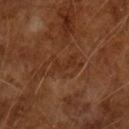Impression:
Part of a total-body skin-imaging series; this lesion was reviewed on a skin check and was not flagged for biopsy.
Image and clinical context:
Cropped from a whole-body photographic skin survey; the tile spans about 15 mm. Automated image analysis of the tile measured a footprint of about 3 mm² and a shape eccentricity near 0.9. The software also gave an automated nevus-likeness rating near 0 out of 100 and lesion-presence confidence of about 80/100. The subject is a male in their mid- to late 60s.